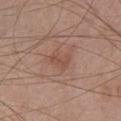No biopsy was performed on this lesion — it was imaged during a full skin examination and was not determined to be concerning. The subject is a female aged around 30. A roughly 15 mm field-of-view crop from a total-body skin photograph. From the head or neck.